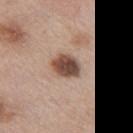Recorded during total-body skin imaging; not selected for excision or biopsy. Cropped from a total-body skin-imaging series; the visible field is about 15 mm. The lesion is located on the left thigh. Imaged with white-light lighting. The lesion-visualizer software estimated an average lesion color of about L≈48 a*≈18 b*≈26 (CIELAB), a lesion–skin lightness drop of about 17, and a normalized lesion–skin contrast near 12. Longest diameter approximately 3.5 mm. The patient is a female approximately 40 years of age.